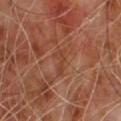{"biopsy_status": "not biopsied; imaged during a skin examination", "lighting": "cross-polarized", "lesion_size": {"long_diameter_mm_approx": 3.5}, "image": {"source": "total-body photography crop", "field_of_view_mm": 15}, "patient": {"sex": "male", "age_approx": 65}}